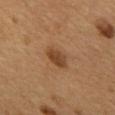Clinical impression:
The lesion was photographed on a routine skin check and not biopsied; there is no pathology result.
Background:
A male patient, approximately 55 years of age. Located on the mid back. This image is a 15 mm lesion crop taken from a total-body photograph.